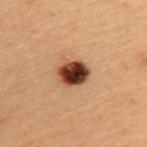Impression: Part of a total-body skin-imaging series; this lesion was reviewed on a skin check and was not flagged for biopsy. Acquisition and patient details: A female patient, aged approximately 40. The lesion is located on the upper back. Cropped from a total-body skin-imaging series; the visible field is about 15 mm. Imaged with cross-polarized lighting. The total-body-photography lesion software estimated a nevus-likeness score of about 100/100 and a detector confidence of about 100 out of 100 that the crop contains a lesion. Longest diameter approximately 3.5 mm.